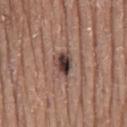biopsy status — total-body-photography surveillance lesion; no biopsy | location — the mid back | lighting — white-light | patient — male, approximately 75 years of age | image source — 15 mm crop, total-body photography | diameter — ~3 mm (longest diameter).